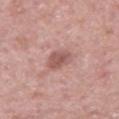Captured during whole-body skin photography for melanoma surveillance; the lesion was not biopsied. A region of skin cropped from a whole-body photographic capture, roughly 15 mm wide. A male patient aged 53 to 57. This is a white-light tile. Longest diameter approximately 3.5 mm. The lesion is on the right upper arm. The total-body-photography lesion software estimated an average lesion color of about L≈55 a*≈23 b*≈23 (CIELAB), a lesion–skin lightness drop of about 10, and a lesion-to-skin contrast of about 7 (normalized; higher = more distinct).